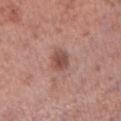The lesion was tiled from a total-body skin photograph and was not biopsied. A male subject, aged approximately 55. The total-body-photography lesion software estimated a lesion area of about 5 mm², an eccentricity of roughly 0.7, and a shape-asymmetry score of about 0.2 (0 = symmetric). And it measured a lesion color around L≈49 a*≈22 b*≈25 in CIELAB. The tile uses white-light illumination. The lesion is located on the leg. Cropped from a whole-body photographic skin survey; the tile spans about 15 mm.